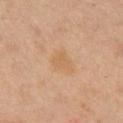Q: Was this lesion biopsied?
A: catalogued during a skin exam; not biopsied
Q: Illumination type?
A: cross-polarized illumination
Q: How large is the lesion?
A: ≈2.5 mm
Q: What is the imaging modality?
A: ~15 mm crop, total-body skin-cancer survey
Q: Lesion location?
A: the arm
Q: Automated lesion metrics?
A: a footprint of about 4 mm² and a symmetry-axis asymmetry near 0.35; a lesion–skin lightness drop of about 6 and a normalized border contrast of about 5
Q: Patient demographics?
A: male, aged 38 to 42